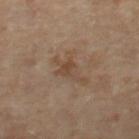Longest diameter approximately 5 mm.
The total-body-photography lesion software estimated a lesion color around L≈44 a*≈15 b*≈27 in CIELAB, about 7 CIELAB-L* units darker than the surrounding skin, and a normalized lesion–skin contrast near 6. It also reported a classifier nevus-likeness of about 0/100 and a detector confidence of about 100 out of 100 that the crop contains a lesion.
A close-up tile cropped from a whole-body skin photograph, about 15 mm across.
The lesion is located on the left thigh.
A female subject, aged approximately 50.
This is a cross-polarized tile.